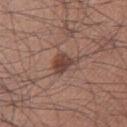<tbp_lesion>
  <biopsy_status>not biopsied; imaged during a skin examination</biopsy_status>
  <site>right thigh</site>
  <lesion_size>
    <long_diameter_mm_approx>3.5</long_diameter_mm_approx>
  </lesion_size>
  <patient>
    <sex>male</sex>
    <age_approx>35</age_approx>
  </patient>
  <image>
    <source>total-body photography crop</source>
    <field_of_view_mm>15</field_of_view_mm>
  </image>
  <lighting>white-light</lighting>
  <automated_metrics>
    <area_mm2_approx>5.5</area_mm2_approx>
    <eccentricity>0.7</eccentricity>
    <nevus_likeness_0_100>75</nevus_likeness_0_100>
    <lesion_detection_confidence_0_100>100</lesion_detection_confidence_0_100>
  </automated_metrics>
</tbp_lesion>